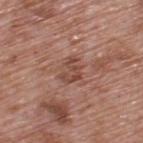Assessment:
The lesion was photographed on a routine skin check and not biopsied; there is no pathology result.
Acquisition and patient details:
A 15 mm crop from a total-body photograph taken for skin-cancer surveillance. The patient is a male in their 70s. Captured under white-light illumination. The lesion is on the back. The lesion's longest dimension is about 3 mm.